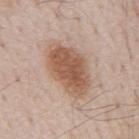The lesion was photographed on a routine skin check and not biopsied; there is no pathology result. A 15 mm crop from a total-body photograph taken for skin-cancer surveillance. A male patient, aged around 80. The lesion is located on the mid back.